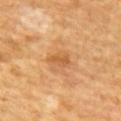Imaged during a routine full-body skin examination; the lesion was not biopsied and no histopathology is available.
Captured under cross-polarized illumination.
The lesion is on the mid back.
A 15 mm crop from a total-body photograph taken for skin-cancer surveillance.
The patient is a male in their 60s.
The lesion's longest dimension is about 2.5 mm.